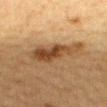Part of a total-body skin-imaging series; this lesion was reviewed on a skin check and was not flagged for biopsy.
Imaged with cross-polarized lighting.
A region of skin cropped from a whole-body photographic capture, roughly 15 mm wide.
From the mid back.
About 7 mm across.
The subject is a female approximately 55 years of age.